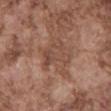Case summary:
• notes: imaged on a skin check; not biopsied
• location: the mid back
• imaging modality: ~15 mm tile from a whole-body skin photo
• subject: male, aged 73–77